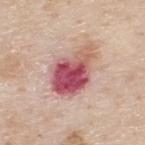Q: Illumination type?
A: white-light illumination
Q: What is the imaging modality?
A: 15 mm crop, total-body photography
Q: Lesion size?
A: ~6.5 mm (longest diameter)
Q: What are the patient's age and sex?
A: male, aged around 80
Q: Lesion location?
A: the upper back
Q: What did automated image analysis measure?
A: a footprint of about 19 mm², an eccentricity of roughly 0.75, and a shape-asymmetry score of about 0.3 (0 = symmetric); a lesion color around L≈55 a*≈30 b*≈21 in CIELAB, about 17 CIELAB-L* units darker than the surrounding skin, and a normalized border contrast of about 11; border irregularity of about 3.5 on a 0–10 scale, internal color variation of about 10 on a 0–10 scale, and radial color variation of about 5